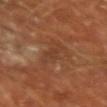Assessment:
Recorded during total-body skin imaging; not selected for excision or biopsy.
Image and clinical context:
The total-body-photography lesion software estimated roughly 6 lightness units darker than nearby skin and a normalized border contrast of about 5.5. The software also gave a color-variation rating of about 2.5/10 and radial color variation of about 1. Captured under cross-polarized illumination. Longest diameter approximately 6.5 mm. The subject is a male aged around 65. From the left forearm. Cropped from a total-body skin-imaging series; the visible field is about 15 mm.